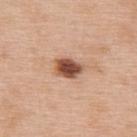Impression: Part of a total-body skin-imaging series; this lesion was reviewed on a skin check and was not flagged for biopsy. Context: This is a white-light tile. An algorithmic analysis of the crop reported a lesion color around L≈50 a*≈24 b*≈31 in CIELAB, about 19 CIELAB-L* units darker than the surrounding skin, and a normalized border contrast of about 12.5. The analysis additionally found peripheral color asymmetry of about 1. A close-up tile cropped from a whole-body skin photograph, about 15 mm across. A male subject roughly 60 years of age. From the upper back. Approximately 3.5 mm at its widest.